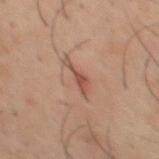biopsy_status: not biopsied; imaged during a skin examination
site: mid back
patient:
  sex: male
  age_approx: 40
automated_metrics:
  cielab_L: 50
  cielab_a: 22
  cielab_b: 27
  vs_skin_darker_L: 10.0
  vs_skin_contrast_norm: 7.0
  color_variation_0_10: 1.5
  nevus_likeness_0_100: 0
  lesion_detection_confidence_0_100: 100
lesion_size:
  long_diameter_mm_approx: 4.5
image:
  source: total-body photography crop
  field_of_view_mm: 15